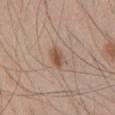Recorded during total-body skin imaging; not selected for excision or biopsy. The patient is a male approximately 45 years of age. Captured under white-light illumination. From the abdomen. Longest diameter approximately 3 mm. A 15 mm close-up extracted from a 3D total-body photography capture.